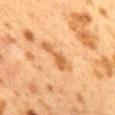Findings:
– workup — no biopsy performed (imaged during a skin exam)
– illumination — cross-polarized
– site — the mid back
– image source — total-body-photography crop, ~15 mm field of view
– subject — female, aged approximately 40